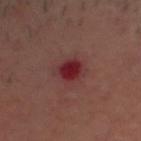Findings:
- follow-up — imaged on a skin check; not biopsied
- image source — total-body-photography crop, ~15 mm field of view
- subject — male, aged around 55
- location — the head or neck
- lesion diameter — ~3 mm (longest diameter)
- illumination — cross-polarized illumination